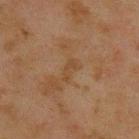notes: catalogued during a skin exam; not biopsied | patient: male, in their mid-40s | site: the upper back | tile lighting: cross-polarized | imaging modality: total-body-photography crop, ~15 mm field of view | lesion diameter: ~2.5 mm (longest diameter).